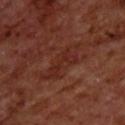Recorded during total-body skin imaging; not selected for excision or biopsy. A male patient, roughly 70 years of age. This image is a 15 mm lesion crop taken from a total-body photograph. On the back.